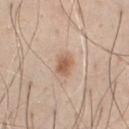workup: imaged on a skin check; not biopsied
site: the chest
subject: male, roughly 45 years of age
image source: 15 mm crop, total-body photography